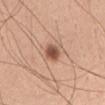{
  "biopsy_status": "not biopsied; imaged during a skin examination",
  "image": {
    "source": "total-body photography crop",
    "field_of_view_mm": 15
  },
  "patient": {
    "sex": "male",
    "age_approx": 40
  },
  "site": "chest"
}